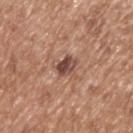biopsy_status: not biopsied; imaged during a skin examination
automated_metrics:
  eccentricity: 0.7
  shape_asymmetry: 0.25
  nevus_likeness_0_100: 0
  lesion_detection_confidence_0_100: 100
image:
  source: total-body photography crop
  field_of_view_mm: 15
lesion_size:
  long_diameter_mm_approx: 3.0
site: upper back
lighting: white-light
patient:
  sex: male
  age_approx: 65